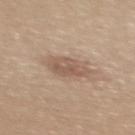No biopsy was performed on this lesion — it was imaged during a full skin examination and was not determined to be concerning. A female patient, aged around 30. The lesion is on the upper back. A region of skin cropped from a whole-body photographic capture, roughly 15 mm wide.